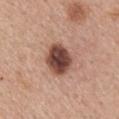<tbp_lesion>
<biopsy_status>not biopsied; imaged during a skin examination</biopsy_status>
<lesion_size>
  <long_diameter_mm_approx>4.0</long_diameter_mm_approx>
</lesion_size>
<lighting>white-light</lighting>
<site>mid back</site>
<image>
  <source>total-body photography crop</source>
  <field_of_view_mm>15</field_of_view_mm>
</image>
<patient>
  <sex>male</sex>
  <age_approx>55</age_approx>
</patient>
<automated_metrics>
  <area_mm2_approx>11.0</area_mm2_approx>
  <eccentricity>0.5</eccentricity>
  <shape_asymmetry>0.1</shape_asymmetry>
  <color_variation_0_10>6.0</color_variation_0_10>
  <peripheral_color_asymmetry>1.5</peripheral_color_asymmetry>
</automated_metrics>
</tbp_lesion>